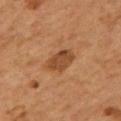The lesion was tiled from a total-body skin photograph and was not biopsied. Longest diameter approximately 4 mm. A 15 mm close-up extracted from a 3D total-body photography capture. From the back. Automated tile analysis of the lesion measured a border-irregularity index near 2/10 and internal color variation of about 2.5 on a 0–10 scale. Imaged with cross-polarized lighting. A male patient, in their mid-50s.